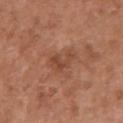This lesion was catalogued during total-body skin photography and was not selected for biopsy. The lesion is on the chest. A 15 mm close-up tile from a total-body photography series done for melanoma screening. The patient is a female aged around 50.